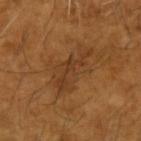The lesion was tiled from a total-body skin photograph and was not biopsied. An algorithmic analysis of the crop reported a lesion area of about 11 mm² and a shape-asymmetry score of about 0.45 (0 = symmetric). The software also gave an average lesion color of about L≈38 a*≈21 b*≈35 (CIELAB), a lesion–skin lightness drop of about 7, and a lesion-to-skin contrast of about 6 (normalized; higher = more distinct). On the left forearm. A roughly 15 mm field-of-view crop from a total-body skin photograph. The lesion's longest dimension is about 6 mm. A male subject aged 63–67. Imaged with cross-polarized lighting.